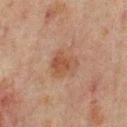Clinical impression: Recorded during total-body skin imaging; not selected for excision or biopsy. Context: From the mid back. This image is a 15 mm lesion crop taken from a total-body photograph. A male subject, about 65 years old.